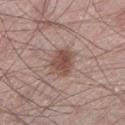This lesion was catalogued during total-body skin photography and was not selected for biopsy. This image is a 15 mm lesion crop taken from a total-body photograph. Located on the leg. A male patient, roughly 65 years of age.